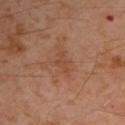workup = catalogued during a skin exam; not biopsied
lesion size = ~2.5 mm (longest diameter)
image = ~15 mm crop, total-body skin-cancer survey
body site = the left upper arm
patient = male, aged 53–57
illumination = cross-polarized illumination